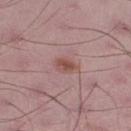Q: Was this lesion biopsied?
A: catalogued during a skin exam; not biopsied
Q: Automated lesion metrics?
A: an area of roughly 4 mm² and an outline eccentricity of about 0.7 (0 = round, 1 = elongated); a normalized border contrast of about 7.5; a border-irregularity rating of about 1.5/10 and radial color variation of about 1
Q: How was the tile lit?
A: white-light
Q: What is the lesion's diameter?
A: ≈2.5 mm
Q: What are the patient's age and sex?
A: male, aged around 50
Q: What is the imaging modality?
A: total-body-photography crop, ~15 mm field of view
Q: What is the anatomic site?
A: the right lower leg